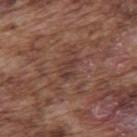<tbp_lesion>
  <biopsy_status>not biopsied; imaged during a skin examination</biopsy_status>
  <patient>
    <sex>male</sex>
    <age_approx>75</age_approx>
  </patient>
  <image>
    <source>total-body photography crop</source>
    <field_of_view_mm>15</field_of_view_mm>
  </image>
  <lighting>white-light</lighting>
  <lesion_size>
    <long_diameter_mm_approx>2.5</long_diameter_mm_approx>
  </lesion_size>
  <site>upper back</site>
</tbp_lesion>